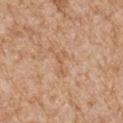The lesion was photographed on a routine skin check and not biopsied; there is no pathology result. The patient is a male about 65 years old. Captured under white-light illumination. The lesion-visualizer software estimated an average lesion color of about L≈59 a*≈20 b*≈35 (CIELAB) and about 6 CIELAB-L* units darker than the surrounding skin. The software also gave a border-irregularity rating of about 6.5/10 and a color-variation rating of about 0/10. The analysis additionally found a nevus-likeness score of about 0/100 and lesion-presence confidence of about 100/100. Located on the arm. Cropped from a total-body skin-imaging series; the visible field is about 15 mm. Longest diameter approximately 3 mm.